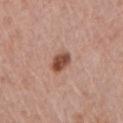Notes:
* workup: total-body-photography surveillance lesion; no biopsy
* tile lighting: white-light illumination
* automated metrics: a lesion area of about 5 mm² and two-axis asymmetry of about 0.15
* acquisition: ~15 mm crop, total-body skin-cancer survey
* subject: male, aged around 70
* location: the chest
* lesion diameter: about 3 mm